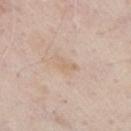{"automated_metrics": {"area_mm2_approx": 3.0, "shape_asymmetry": 0.45, "cielab_L": 66, "cielab_a": 15, "cielab_b": 30, "vs_skin_darker_L": 5.0, "vs_skin_contrast_norm": 4.5}, "patient": {"sex": "male", "age_approx": 70}, "lighting": "white-light", "site": "right upper arm", "lesion_size": {"long_diameter_mm_approx": 2.5}, "image": {"source": "total-body photography crop", "field_of_view_mm": 15}}